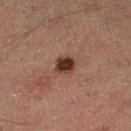diameter: ≈2.5 mm; image source: ~15 mm crop, total-body skin-cancer survey; tile lighting: cross-polarized; anatomic site: the left lower leg; subject: male, aged 48 to 52.